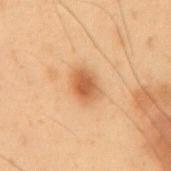This lesion was catalogued during total-body skin photography and was not selected for biopsy. A close-up tile cropped from a whole-body skin photograph, about 15 mm across. The lesion is on the back. Captured under cross-polarized illumination. A male patient approximately 55 years of age. The recorded lesion diameter is about 3.5 mm. An algorithmic analysis of the crop reported a lesion color around L≈48 a*≈20 b*≈34 in CIELAB and a lesion-to-skin contrast of about 8 (normalized; higher = more distinct). The software also gave a border-irregularity index near 2/10, a color-variation rating of about 3/10, and peripheral color asymmetry of about 1. The analysis additionally found a nevus-likeness score of about 100/100 and lesion-presence confidence of about 100/100.